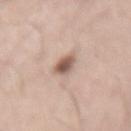workup: imaged on a skin check; not biopsied
diameter: ~2.5 mm (longest diameter)
site: the right forearm
subject: male, aged around 60
tile lighting: white-light illumination
image-analysis metrics: a footprint of about 5 mm², an eccentricity of roughly 0.4, and a shape-asymmetry score of about 0.25 (0 = symmetric); border irregularity of about 2 on a 0–10 scale, internal color variation of about 5 on a 0–10 scale, and peripheral color asymmetry of about 1.5
image: total-body-photography crop, ~15 mm field of view